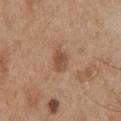follow-up: total-body-photography surveillance lesion; no biopsy
lighting: white-light illumination
acquisition: 15 mm crop, total-body photography
patient: male, roughly 55 years of age
size: about 2.5 mm
automated lesion analysis: a lesion color around L≈50 a*≈20 b*≈32 in CIELAB, roughly 9 lightness units darker than nearby skin, and a normalized border contrast of about 7; a border-irregularity index near 1.5/10, internal color variation of about 2.5 on a 0–10 scale, and a peripheral color-asymmetry measure near 1; a nevus-likeness score of about 40/100 and lesion-presence confidence of about 100/100
body site: the chest